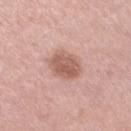Captured during whole-body skin photography for melanoma surveillance; the lesion was not biopsied. The tile uses white-light illumination. Cropped from a whole-body photographic skin survey; the tile spans about 15 mm. The subject is a female approximately 35 years of age. Approximately 4.5 mm at its widest. The lesion is located on the arm. Automated image analysis of the tile measured a footprint of about 10 mm², an outline eccentricity of about 0.8 (0 = round, 1 = elongated), and two-axis asymmetry of about 0.15. The software also gave an average lesion color of about L≈58 a*≈22 b*≈25 (CIELAB), about 12 CIELAB-L* units darker than the surrounding skin, and a normalized border contrast of about 7.5. And it measured a peripheral color-asymmetry measure near 1. The analysis additionally found an automated nevus-likeness rating near 45 out of 100 and a lesion-detection confidence of about 100/100.